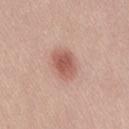A roughly 15 mm field-of-view crop from a total-body skin photograph. The lesion is located on the lower back. A female patient in their 20s.